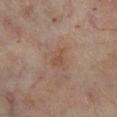This lesion was catalogued during total-body skin photography and was not selected for biopsy. A male patient roughly 70 years of age. Located on the right lower leg. A close-up tile cropped from a whole-body skin photograph, about 15 mm across. Measured at roughly 2.5 mm in maximum diameter. This is a cross-polarized tile.